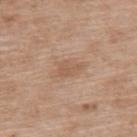Q: Where on the body is the lesion?
A: the upper back
Q: What did automated image analysis measure?
A: an area of roughly 4 mm² and an outline eccentricity of about 0.7 (0 = round, 1 = elongated); a mean CIELAB color near L≈56 a*≈19 b*≈31 and roughly 7 lightness units darker than nearby skin; a within-lesion color-variation index near 2/10 and radial color variation of about 0.5; an automated nevus-likeness rating near 0 out of 100 and a detector confidence of about 100 out of 100 that the crop contains a lesion
Q: Who is the patient?
A: female, aged 73 to 77
Q: What kind of image is this?
A: ~15 mm tile from a whole-body skin photo
Q: How was the tile lit?
A: white-light